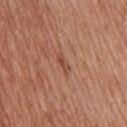Part of a total-body skin-imaging series; this lesion was reviewed on a skin check and was not flagged for biopsy. The lesion is located on the upper back. Approximately 2.5 mm at its widest. A 15 mm close-up extracted from a 3D total-body photography capture. The lesion-visualizer software estimated a mean CIELAB color near L≈48 a*≈24 b*≈29 and a normalized border contrast of about 6. The analysis additionally found border irregularity of about 4 on a 0–10 scale, a within-lesion color-variation index near 0/10, and a peripheral color-asymmetry measure near 0. A male subject aged approximately 70. Captured under white-light illumination.